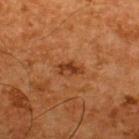Part of a total-body skin-imaging series; this lesion was reviewed on a skin check and was not flagged for biopsy.
Imaged with cross-polarized lighting.
The lesion is on the back.
The patient is a male in their mid-60s.
Longest diameter approximately 3 mm.
Cropped from a total-body skin-imaging series; the visible field is about 15 mm.